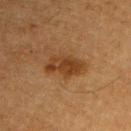follow-up: catalogued during a skin exam; not biopsied | image: 15 mm crop, total-body photography | body site: the right upper arm | subject: male, in their mid-70s.